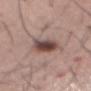Clinical impression:
The lesion was photographed on a routine skin check and not biopsied; there is no pathology result.
Acquisition and patient details:
A male subject, about 55 years old. This image is a 15 mm lesion crop taken from a total-body photograph. The lesion's longest dimension is about 4.5 mm. Located on the abdomen. Imaged with white-light lighting.